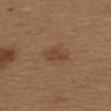notes = no biopsy performed (imaged during a skin exam) | lesion size = ~3.5 mm (longest diameter) | patient = female, aged 38–42 | lighting = white-light | location = the upper back | acquisition = total-body-photography crop, ~15 mm field of view | automated metrics = a lesion color around L≈43 a*≈18 b*≈30 in CIELAB, a lesion–skin lightness drop of about 7, and a normalized lesion–skin contrast near 6; a classifier nevus-likeness of about 25/100.